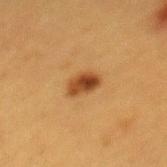Recorded during total-body skin imaging; not selected for excision or biopsy. Cropped from a whole-body photographic skin survey; the tile spans about 15 mm. Located on the mid back. The subject is a female about 40 years old. The lesion's longest dimension is about 3.5 mm. An algorithmic analysis of the crop reported a mean CIELAB color near L≈38 a*≈20 b*≈34, a lesion–skin lightness drop of about 12, and a lesion-to-skin contrast of about 10 (normalized; higher = more distinct). It also reported a nevus-likeness score of about 100/100 and a lesion-detection confidence of about 100/100.